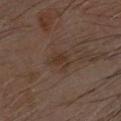The lesion was photographed on a routine skin check and not biopsied; there is no pathology result. The tile uses cross-polarized illumination. The lesion is located on the head or neck. A lesion tile, about 15 mm wide, cut from a 3D total-body photograph. The subject is a male about 70 years old.